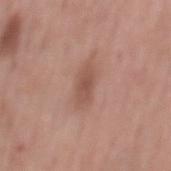notes: imaged on a skin check; not biopsied
diameter: about 4 mm
TBP lesion metrics: an average lesion color of about L≈52 a*≈21 b*≈26 (CIELAB) and a lesion-to-skin contrast of about 6.5 (normalized; higher = more distinct)
lighting: white-light
patient: male, aged around 55
body site: the mid back
image source: ~15 mm crop, total-body skin-cancer survey